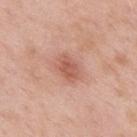This lesion was catalogued during total-body skin photography and was not selected for biopsy. Longest diameter approximately 3 mm. This is a white-light tile. A 15 mm close-up tile from a total-body photography series done for melanoma screening. A male patient, aged 53 to 57. Located on the back. Automated tile analysis of the lesion measured an automated nevus-likeness rating near 45 out of 100.